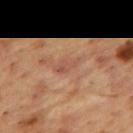Clinical impression: Recorded during total-body skin imaging; not selected for excision or biopsy. Acquisition and patient details: Automated image analysis of the tile measured a shape eccentricity near 0.9 and two-axis asymmetry of about 0.35. The software also gave a border-irregularity rating of about 4/10, internal color variation of about 1.5 on a 0–10 scale, and radial color variation of about 0.5. The analysis additionally found an automated nevus-likeness rating near 0 out of 100 and a lesion-detection confidence of about 90/100. Cropped from a total-body skin-imaging series; the visible field is about 15 mm. The tile uses cross-polarized illumination. The patient is a male aged approximately 55. The lesion is located on the upper back. Measured at roughly 3 mm in maximum diameter.